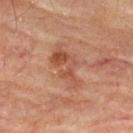Findings:
– follow-up: imaged on a skin check; not biopsied
– site: the upper back
– automated metrics: an area of roughly 11 mm² and a shape eccentricity near 0.85
– subject: male, aged approximately 75
– image: 15 mm crop, total-body photography
– illumination: cross-polarized